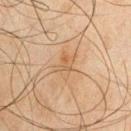Impression:
No biopsy was performed on this lesion — it was imaged during a full skin examination and was not determined to be concerning.
Acquisition and patient details:
A male patient about 45 years old. A lesion tile, about 15 mm wide, cut from a 3D total-body photograph. On the chest.